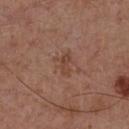Captured during whole-body skin photography for melanoma surveillance; the lesion was not biopsied. This is a white-light tile. A 15 mm close-up extracted from a 3D total-body photography capture. A male subject aged around 55. The recorded lesion diameter is about 3 mm. Located on the front of the torso. The total-body-photography lesion software estimated an area of roughly 4 mm². The software also gave a border-irregularity rating of about 5/10, a color-variation rating of about 2/10, and a peripheral color-asymmetry measure near 0.5.